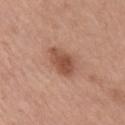Impression:
The lesion was photographed on a routine skin check and not biopsied; there is no pathology result.
Image and clinical context:
A lesion tile, about 15 mm wide, cut from a 3D total-body photograph. Located on the right upper arm. A male patient, about 55 years old. Automated image analysis of the tile measured a lesion area of about 7.5 mm². It also reported a mean CIELAB color near L≈51 a*≈23 b*≈30, roughly 11 lightness units darker than nearby skin, and a normalized lesion–skin contrast near 8. And it measured a detector confidence of about 100 out of 100 that the crop contains a lesion. About 4 mm across. This is a white-light tile.